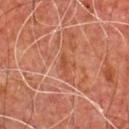The lesion was photographed on a routine skin check and not biopsied; there is no pathology result. The lesion is located on the chest. Cropped from a whole-body photographic skin survey; the tile spans about 15 mm. The recorded lesion diameter is about 2.5 mm. The subject is a male aged 63–67.